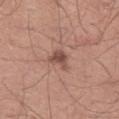The lesion was tiled from a total-body skin photograph and was not biopsied.
The lesion is on the left thigh.
The lesion's longest dimension is about 2.5 mm.
A male patient, aged 38 to 42.
A roughly 15 mm field-of-view crop from a total-body skin photograph.